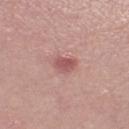Q: Is there a histopathology result?
A: no biopsy performed (imaged during a skin exam)
Q: What is the imaging modality?
A: 15 mm crop, total-body photography
Q: How was the tile lit?
A: white-light
Q: Who is the patient?
A: female, about 50 years old
Q: Where on the body is the lesion?
A: the leg
Q: How large is the lesion?
A: ≈2.5 mm